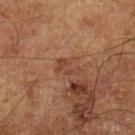The lesion was tiled from a total-body skin photograph and was not biopsied.
This is a cross-polarized tile.
About 3 mm across.
A 15 mm close-up tile from a total-body photography series done for melanoma screening.
A male patient, aged approximately 65.